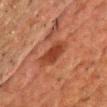Assessment: This lesion was catalogued during total-body skin photography and was not selected for biopsy. Context: An algorithmic analysis of the crop reported an area of roughly 8 mm², a shape eccentricity near 0.85, and a symmetry-axis asymmetry near 0.25. The analysis additionally found a border-irregularity rating of about 2.5/10, a within-lesion color-variation index near 2.5/10, and a peripheral color-asymmetry measure near 1. And it measured a detector confidence of about 100 out of 100 that the crop contains a lesion. Located on the chest. Cropped from a total-body skin-imaging series; the visible field is about 15 mm. The tile uses cross-polarized illumination. A male subject aged 58 to 62. The recorded lesion diameter is about 4.5 mm.